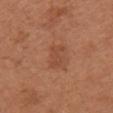workup: no biopsy performed (imaged during a skin exam)
image: total-body-photography crop, ~15 mm field of view
automated metrics: roughly 6 lightness units darker than nearby skin and a normalized lesion–skin contrast near 5; an automated nevus-likeness rating near 10 out of 100 and a detector confidence of about 100 out of 100 that the crop contains a lesion
anatomic site: the chest
lesion diameter: ≈3 mm
subject: female, approximately 65 years of age
tile lighting: white-light illumination